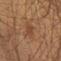Notes:
– notes: imaged on a skin check; not biopsied
– acquisition: ~15 mm tile from a whole-body skin photo
– tile lighting: cross-polarized
– anatomic site: the right upper arm
– lesion size: ≈4 mm
– patient: male, in their mid- to late 40s
– TBP lesion metrics: a lesion color around L≈34 a*≈20 b*≈26 in CIELAB, roughly 6 lightness units darker than nearby skin, and a normalized lesion–skin contrast near 5.5; a border-irregularity rating of about 5.5/10, internal color variation of about 0 on a 0–10 scale, and a peripheral color-asymmetry measure near 0